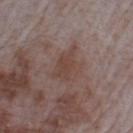{
  "biopsy_status": "not biopsied; imaged during a skin examination",
  "automated_metrics": {
    "area_mm2_approx": 8.5,
    "eccentricity": 0.75,
    "shape_asymmetry": 0.3,
    "color_variation_0_10": 2.0,
    "nevus_likeness_0_100": 0,
    "lesion_detection_confidence_0_100": 100
  },
  "lesion_size": {
    "long_diameter_mm_approx": 4.5
  },
  "image": {
    "source": "total-body photography crop",
    "field_of_view_mm": 15
  },
  "site": "left upper arm",
  "lighting": "white-light",
  "patient": {
    "sex": "male",
    "age_approx": 65
  }
}